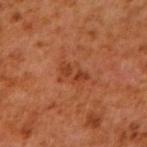Imaged during a routine full-body skin examination; the lesion was not biopsied and no histopathology is available. Located on the right upper arm. Approximately 3.5 mm at its widest. The subject is a male roughly 60 years of age. A region of skin cropped from a whole-body photographic capture, roughly 15 mm wide. The tile uses cross-polarized illumination. The total-body-photography lesion software estimated an area of roughly 5.5 mm², an eccentricity of roughly 0.8, and two-axis asymmetry of about 0.4. And it measured an automated nevus-likeness rating near 0 out of 100 and a lesion-detection confidence of about 100/100.